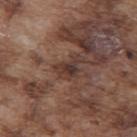Impression: Captured during whole-body skin photography for melanoma surveillance; the lesion was not biopsied.